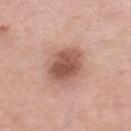Impression:
Part of a total-body skin-imaging series; this lesion was reviewed on a skin check and was not flagged for biopsy.
Image and clinical context:
Captured under white-light illumination. From the left thigh. The lesion's longest dimension is about 4.5 mm. A region of skin cropped from a whole-body photographic capture, roughly 15 mm wide. A female subject in their mid-50s.